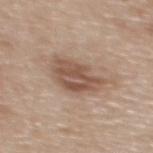follow-up = imaged on a skin check; not biopsied
anatomic site = the back
subject = male, about 70 years old
acquisition = 15 mm crop, total-body photography